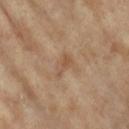From the leg. This image is a 15 mm lesion crop taken from a total-body photograph. An algorithmic analysis of the crop reported a mean CIELAB color near L≈52 a*≈17 b*≈31, roughly 7 lightness units darker than nearby skin, and a lesion-to-skin contrast of about 5.5 (normalized; higher = more distinct). It also reported a border-irregularity rating of about 4.5/10, internal color variation of about 1.5 on a 0–10 scale, and a peripheral color-asymmetry measure near 0.5. A female subject, approximately 75 years of age. Approximately 3 mm at its widest.